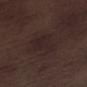Q: Was this lesion biopsied?
A: catalogued during a skin exam; not biopsied
Q: What lighting was used for the tile?
A: white-light illumination
Q: What are the patient's age and sex?
A: male, roughly 70 years of age
Q: How was this image acquired?
A: ~15 mm tile from a whole-body skin photo
Q: What is the lesion's diameter?
A: ~5 mm (longest diameter)
Q: What is the anatomic site?
A: the right lower leg